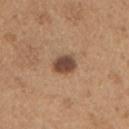The lesion is located on the chest. The patient is a male aged approximately 65. A region of skin cropped from a whole-body photographic capture, roughly 15 mm wide. Automated tile analysis of the lesion measured an average lesion color of about L≈46 a*≈19 b*≈28 (CIELAB), about 15 CIELAB-L* units darker than the surrounding skin, and a normalized lesion–skin contrast near 11. And it measured a border-irregularity rating of about 1.5/10 and radial color variation of about 1. Imaged with white-light lighting. Measured at roughly 3 mm in maximum diameter.